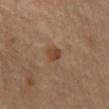* follow-up · imaged on a skin check; not biopsied
* patient · female, aged around 65
* illumination · cross-polarized
* lesion size · about 2 mm
* acquisition · total-body-photography crop, ~15 mm field of view
* location · the chest